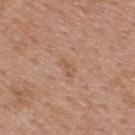Recorded during total-body skin imaging; not selected for excision or biopsy. Imaged with white-light lighting. The recorded lesion diameter is about 2.5 mm. A male subject aged 53 to 57. Automated tile analysis of the lesion measured an outline eccentricity of about 0.85 (0 = round, 1 = elongated) and a symmetry-axis asymmetry near 0.35. It also reported a lesion-detection confidence of about 100/100. Located on the upper back. A 15 mm close-up tile from a total-body photography series done for melanoma screening.